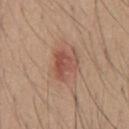Clinical impression:
Part of a total-body skin-imaging series; this lesion was reviewed on a skin check and was not flagged for biopsy.
Image and clinical context:
The tile uses white-light illumination. Located on the chest. The subject is a male approximately 20 years of age. A 15 mm close-up extracted from a 3D total-body photography capture. About 3.5 mm across.